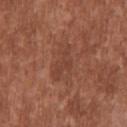Captured during whole-body skin photography for melanoma surveillance; the lesion was not biopsied.